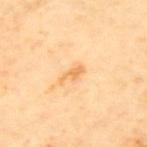follow-up: total-body-photography surveillance lesion; no biopsy
automated lesion analysis: an area of roughly 2.5 mm², an eccentricity of roughly 0.9, and a shape-asymmetry score of about 0.4 (0 = symmetric); a classifier nevus-likeness of about 0/100 and lesion-presence confidence of about 100/100
imaging modality: total-body-photography crop, ~15 mm field of view
location: the upper back
tile lighting: cross-polarized
lesion diameter: ~2.5 mm (longest diameter)
subject: male, roughly 65 years of age